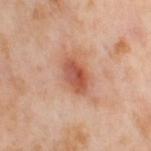Assessment: Part of a total-body skin-imaging series; this lesion was reviewed on a skin check and was not flagged for biopsy.